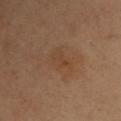Notes:
• biopsy status: total-body-photography surveillance lesion; no biopsy
• size: ≈3.5 mm
• image source: total-body-photography crop, ~15 mm field of view
• anatomic site: the arm
• automated lesion analysis: border irregularity of about 4 on a 0–10 scale
• illumination: cross-polarized illumination
• patient: female, aged approximately 35